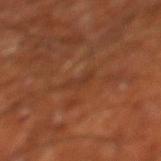Findings:
• follow-up · imaged on a skin check; not biopsied
• anatomic site · the left lower leg
• lesion diameter · about 3 mm
• image · ~15 mm crop, total-body skin-cancer survey
• automated metrics · a footprint of about 2.5 mm² and a shape-asymmetry score of about 0.4 (0 = symmetric); a border-irregularity rating of about 5.5/10, internal color variation of about 0 on a 0–10 scale, and radial color variation of about 0
• patient · male, about 65 years old
• lighting · cross-polarized